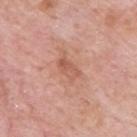Captured during whole-body skin photography for melanoma surveillance; the lesion was not biopsied.
A lesion tile, about 15 mm wide, cut from a 3D total-body photograph.
Automated tile analysis of the lesion measured a lesion area of about 4 mm², an eccentricity of roughly 0.85, and two-axis asymmetry of about 0.3. The analysis additionally found border irregularity of about 3 on a 0–10 scale and a color-variation rating of about 4.5/10.
Captured under white-light illumination.
Approximately 3 mm at its widest.
From the back.
A male subject, in their mid-50s.